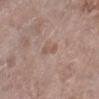biopsy status: total-body-photography surveillance lesion; no biopsy | subject: female, about 75 years old | image source: total-body-photography crop, ~15 mm field of view | lighting: white-light illumination | image-analysis metrics: an area of roughly 2.5 mm² and an outline eccentricity of about 0.95 (0 = round, 1 = elongated); a lesion color around L≈54 a*≈18 b*≈25 in CIELAB, roughly 7 lightness units darker than nearby skin, and a normalized lesion–skin contrast near 5.5 | anatomic site: the left lower leg | lesion diameter: about 2.5 mm.